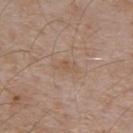* biopsy status · imaged on a skin check; not biopsied
* lesion size · about 3 mm
* body site · the upper back
* patient · male, approximately 55 years of age
* automated lesion analysis · a shape eccentricity near 0.85 and a symmetry-axis asymmetry near 0.35; a lesion color around L≈54 a*≈16 b*≈29 in CIELAB, roughly 5 lightness units darker than nearby skin, and a lesion-to-skin contrast of about 5 (normalized; higher = more distinct)
* acquisition · total-body-photography crop, ~15 mm field of view